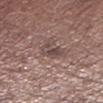| feature | finding |
|---|---|
| workup | no biopsy performed (imaged during a skin exam) |
| tile lighting | white-light illumination |
| patient | male, aged 53–57 |
| location | the left lower leg |
| automated lesion analysis | a lesion color around L≈45 a*≈16 b*≈18 in CIELAB, roughly 8 lightness units darker than nearby skin, and a normalized lesion–skin contrast near 7 |
| image source | ~15 mm crop, total-body skin-cancer survey |
| lesion diameter | ~2.5 mm (longest diameter) |